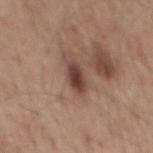Q: Was a biopsy performed?
A: no biopsy performed (imaged during a skin exam)
Q: Automated lesion metrics?
A: a mean CIELAB color near L≈42 a*≈19 b*≈23, roughly 13 lightness units darker than nearby skin, and a normalized lesion–skin contrast near 10; a border-irregularity index near 2.5/10 and peripheral color asymmetry of about 1; a nevus-likeness score of about 100/100 and a detector confidence of about 100 out of 100 that the crop contains a lesion
Q: Illumination type?
A: white-light illumination
Q: Lesion location?
A: the mid back
Q: How was this image acquired?
A: total-body-photography crop, ~15 mm field of view
Q: Who is the patient?
A: male, aged around 55
Q: Lesion size?
A: about 3.5 mm